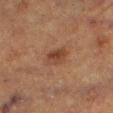No biopsy was performed on this lesion — it was imaged during a full skin examination and was not determined to be concerning. Cropped from a total-body skin-imaging series; the visible field is about 15 mm. Approximately 2.5 mm at its widest. The total-body-photography lesion software estimated a lesion area of about 5 mm², an outline eccentricity of about 0.7 (0 = round, 1 = elongated), and two-axis asymmetry of about 0.2. The analysis additionally found an average lesion color of about L≈33 a*≈18 b*≈26 (CIELAB), roughly 7 lightness units darker than nearby skin, and a normalized lesion–skin contrast near 7. Located on the right thigh. This is a cross-polarized tile. A male patient aged approximately 75.